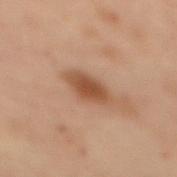Q: Was a biopsy performed?
A: total-body-photography surveillance lesion; no biopsy
Q: Where on the body is the lesion?
A: the upper back
Q: Patient demographics?
A: female, aged 53–57
Q: Illumination type?
A: cross-polarized illumination
Q: How large is the lesion?
A: about 4 mm
Q: How was this image acquired?
A: ~15 mm tile from a whole-body skin photo
Q: Automated lesion metrics?
A: a footprint of about 7.5 mm², an eccentricity of roughly 0.8, and a symmetry-axis asymmetry near 0.2; a lesion color around L≈42 a*≈18 b*≈28 in CIELAB, a lesion–skin lightness drop of about 10, and a normalized lesion–skin contrast near 8